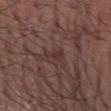workup: no biopsy performed (imaged during a skin exam) | TBP lesion metrics: a lesion area of about 2.5 mm², an outline eccentricity of about 0.9 (0 = round, 1 = elongated), and two-axis asymmetry of about 0.55; internal color variation of about 0.5 on a 0–10 scale; an automated nevus-likeness rating near 0 out of 100 and a detector confidence of about 60 out of 100 that the crop contains a lesion | anatomic site: the arm | subject: male, about 70 years old | lighting: white-light | lesion size: ~3 mm (longest diameter) | image: 15 mm crop, total-body photography.